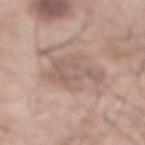Clinical impression:
No biopsy was performed on this lesion — it was imaged during a full skin examination and was not determined to be concerning.
Image and clinical context:
A male subject in their 70s. The lesion's longest dimension is about 5 mm. Located on the front of the torso. Captured under white-light illumination. A 15 mm crop from a total-body photograph taken for skin-cancer surveillance.